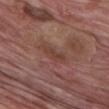Q: Is there a histopathology result?
A: catalogued during a skin exam; not biopsied
Q: How was this image acquired?
A: ~15 mm tile from a whole-body skin photo
Q: Patient demographics?
A: male, in their mid-60s
Q: Automated lesion metrics?
A: a lesion area of about 3.5 mm² and two-axis asymmetry of about 0.35; a mean CIELAB color near L≈40 a*≈21 b*≈25, about 7 CIELAB-L* units darker than the surrounding skin, and a lesion-to-skin contrast of about 6 (normalized; higher = more distinct); a within-lesion color-variation index near 0.5/10 and radial color variation of about 0
Q: What is the anatomic site?
A: the chest
Q: What lighting was used for the tile?
A: white-light illumination